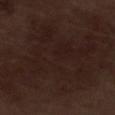No biopsy was performed on this lesion — it was imaged during a full skin examination and was not determined to be concerning.
Captured under white-light illumination.
The lesion is located on the leg.
A 15 mm close-up tile from a total-body photography series done for melanoma screening.
A male patient, aged around 70.
The lesion's longest dimension is about 15 mm.